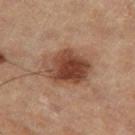Impression: Part of a total-body skin-imaging series; this lesion was reviewed on a skin check and was not flagged for biopsy. Context: A 15 mm crop from a total-body photograph taken for skin-cancer surveillance. Located on the left thigh. A male subject aged approximately 85 or older.